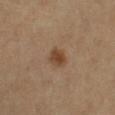{"biopsy_status": "not biopsied; imaged during a skin examination", "automated_metrics": {"cielab_L": 43, "cielab_a": 18, "cielab_b": 31, "vs_skin_darker_L": 10.0, "vs_skin_contrast_norm": 8.5}, "site": "leg", "lesion_size": {"long_diameter_mm_approx": 2.5}, "patient": {"sex": "female", "age_approx": 55}, "image": {"source": "total-body photography crop", "field_of_view_mm": 15}, "lighting": "cross-polarized"}